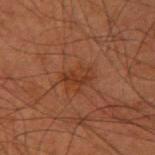biopsy status — no biopsy performed (imaged during a skin exam)
diameter — about 3 mm
illumination — cross-polarized
subject — male, approximately 60 years of age
image source — 15 mm crop, total-body photography
image-analysis metrics — an area of roughly 5 mm² and a symmetry-axis asymmetry near 0.3; roughly 5 lightness units darker than nearby skin; a color-variation rating of about 2/10
site — the right upper arm